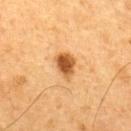{
  "biopsy_status": "not biopsied; imaged during a skin examination",
  "automated_metrics": {
    "eccentricity": 0.65,
    "shape_asymmetry": 0.25,
    "cielab_L": 47,
    "cielab_a": 21,
    "cielab_b": 38,
    "vs_skin_darker_L": 14.0,
    "vs_skin_contrast_norm": 10.0
  },
  "image": {
    "source": "total-body photography crop",
    "field_of_view_mm": 15
  },
  "lesion_size": {
    "long_diameter_mm_approx": 3.5
  },
  "patient": {
    "sex": "male",
    "age_approx": 65
  },
  "site": "back",
  "lighting": "cross-polarized"
}